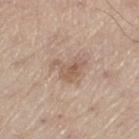notes: total-body-photography surveillance lesion; no biopsy | TBP lesion metrics: a lesion area of about 6.5 mm² and an outline eccentricity of about 0.7 (0 = round, 1 = elongated); roughly 9 lightness units darker than nearby skin and a normalized border contrast of about 6; a border-irregularity rating of about 6/10 and a color-variation rating of about 3.5/10 | body site: the left thigh | tile lighting: white-light | patient: male, approximately 70 years of age | image source: ~15 mm crop, total-body skin-cancer survey | diameter: ≈4 mm.